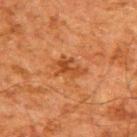A male subject aged 58–62.
Automated image analysis of the tile measured an eccentricity of roughly 0.85 and a symmetry-axis asymmetry near 0.4. The analysis additionally found a lesion color around L≈38 a*≈24 b*≈34 in CIELAB, about 7 CIELAB-L* units darker than the surrounding skin, and a normalized border contrast of about 6.5. The analysis additionally found border irregularity of about 5.5 on a 0–10 scale, a within-lesion color-variation index near 3/10, and a peripheral color-asymmetry measure near 1.
The lesion's longest dimension is about 4 mm.
A 15 mm crop from a total-body photograph taken for skin-cancer surveillance.
The lesion is located on the upper back.
The tile uses cross-polarized illumination.